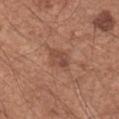| feature | finding |
|---|---|
| notes | total-body-photography surveillance lesion; no biopsy |
| acquisition | ~15 mm tile from a whole-body skin photo |
| location | the arm |
| subject | male, roughly 55 years of age |
| automated lesion analysis | a footprint of about 5.5 mm², an outline eccentricity of about 0.7 (0 = round, 1 = elongated), and a shape-asymmetry score of about 0.25 (0 = symmetric); about 8 CIELAB-L* units darker than the surrounding skin; border irregularity of about 2.5 on a 0–10 scale and radial color variation of about 1.5; an automated nevus-likeness rating near 0 out of 100 and lesion-presence confidence of about 100/100 |
| size | about 3 mm |
| lighting | white-light |